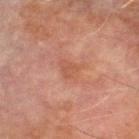biopsy status — no biopsy performed (imaged during a skin exam)
lesion diameter — ~2.5 mm (longest diameter)
imaging modality — 15 mm crop, total-body photography
tile lighting — cross-polarized illumination
subject — male, aged around 70
site — the right lower leg
image-analysis metrics — a lesion area of about 4.5 mm², an eccentricity of roughly 0.55, and a symmetry-axis asymmetry near 0.3; a border-irregularity rating of about 3.5/10 and a within-lesion color-variation index near 1.5/10